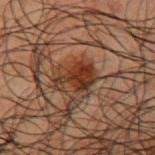follow-up — no biopsy performed (imaged during a skin exam)
automated metrics — a lesion area of about 8 mm², an outline eccentricity of about 0.85 (0 = round, 1 = elongated), and a symmetry-axis asymmetry near 0.45; border irregularity of about 5 on a 0–10 scale, a color-variation rating of about 3/10, and peripheral color asymmetry of about 1
acquisition — total-body-photography crop, ~15 mm field of view
subject — male, aged 48–52
diameter — about 4.5 mm
anatomic site — the left upper arm
lighting — cross-polarized